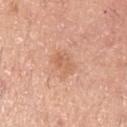| feature | finding |
|---|---|
| notes | catalogued during a skin exam; not biopsied |
| image | ~15 mm crop, total-body skin-cancer survey |
| anatomic site | the left upper arm |
| image-analysis metrics | a lesion area of about 4 mm² and a symmetry-axis asymmetry near 0.25; a lesion color around L≈62 a*≈23 b*≈34 in CIELAB, about 7 CIELAB-L* units darker than the surrounding skin, and a lesion-to-skin contrast of about 5 (normalized; higher = more distinct) |
| subject | male, aged 38 to 42 |
| tile lighting | white-light illumination |
| lesion diameter | ≈2.5 mm |